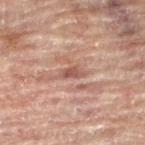workup = imaged on a skin check; not biopsied | subject = female, aged approximately 80 | anatomic site = the right leg | tile lighting = cross-polarized illumination | image = ~15 mm tile from a whole-body skin photo | size = about 2.5 mm.